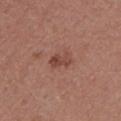Captured during whole-body skin photography for melanoma surveillance; the lesion was not biopsied. The recorded lesion diameter is about 3 mm. The lesion is located on the right upper arm. This is a white-light tile. This image is a 15 mm lesion crop taken from a total-body photograph. A male subject about 40 years old.